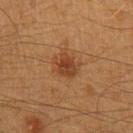Part of a total-body skin-imaging series; this lesion was reviewed on a skin check and was not flagged for biopsy. Located on the left upper arm. The recorded lesion diameter is about 3 mm. A close-up tile cropped from a whole-body skin photograph, about 15 mm across. A male subject, aged approximately 40. The lesion-visualizer software estimated roughly 9 lightness units darker than nearby skin and a normalized lesion–skin contrast near 8. The software also gave border irregularity of about 2.5 on a 0–10 scale, a within-lesion color-variation index near 3/10, and radial color variation of about 1. Captured under cross-polarized illumination.